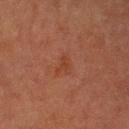Q: Was a biopsy performed?
A: catalogued during a skin exam; not biopsied
Q: What is the imaging modality?
A: total-body-photography crop, ~15 mm field of view
Q: Patient demographics?
A: male, about 80 years old
Q: How large is the lesion?
A: about 2.5 mm
Q: Where on the body is the lesion?
A: the left upper arm
Q: Illumination type?
A: cross-polarized illumination
Q: What did automated image analysis measure?
A: an outline eccentricity of about 0.75 (0 = round, 1 = elongated) and a symmetry-axis asymmetry near 0.45; an average lesion color of about L≈32 a*≈21 b*≈26 (CIELAB), a lesion–skin lightness drop of about 4, and a lesion-to-skin contrast of about 5 (normalized; higher = more distinct); a within-lesion color-variation index near 1.5/10